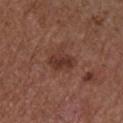No biopsy was performed on this lesion — it was imaged during a full skin examination and was not determined to be concerning.
The lesion-visualizer software estimated an area of roughly 7.5 mm², an outline eccentricity of about 0.65 (0 = round, 1 = elongated), and a symmetry-axis asymmetry near 0.2. The software also gave a lesion-to-skin contrast of about 7.5 (normalized; higher = more distinct). The software also gave a border-irregularity index near 2/10, a color-variation rating of about 3/10, and radial color variation of about 1.
Longest diameter approximately 3.5 mm.
Cropped from a total-body skin-imaging series; the visible field is about 15 mm.
A male subject in their mid-50s.
The lesion is on the arm.
Captured under white-light illumination.